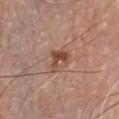Assessment: The lesion was tiled from a total-body skin photograph and was not biopsied. Image and clinical context: Longest diameter approximately 3 mm. A close-up tile cropped from a whole-body skin photograph, about 15 mm across. Captured under white-light illumination. Automated tile analysis of the lesion measured a shape eccentricity near 0.6 and a shape-asymmetry score of about 0.3 (0 = symmetric). It also reported a mean CIELAB color near L≈49 a*≈21 b*≈29, about 10 CIELAB-L* units darker than the surrounding skin, and a normalized border contrast of about 7.5. The lesion is located on the chest. A male patient, aged around 70.